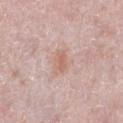Findings:
- body site: the leg
- imaging modality: ~15 mm tile from a whole-body skin photo
- image-analysis metrics: an outline eccentricity of about 0.65 (0 = round, 1 = elongated) and a shape-asymmetry score of about 0.3 (0 = symmetric)
- patient: female, in their 40s
- illumination: white-light illumination
- size: about 2.5 mm